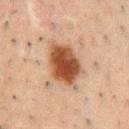workup: no biopsy performed (imaged during a skin exam); location: the chest; lesion size: about 5.5 mm; illumination: cross-polarized illumination; imaging modality: total-body-photography crop, ~15 mm field of view; subject: male, roughly 50 years of age.